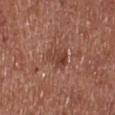Findings:
• automated metrics — a classifier nevus-likeness of about 5/100 and lesion-presence confidence of about 100/100
• lesion diameter — ~3.5 mm (longest diameter)
• subject — male, aged approximately 75
• image source — 15 mm crop, total-body photography
• lighting — white-light
• location — the left lower leg